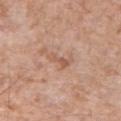Captured during whole-body skin photography for melanoma surveillance; the lesion was not biopsied.
A roughly 15 mm field-of-view crop from a total-body skin photograph.
An algorithmic analysis of the crop reported a footprint of about 3.5 mm², an outline eccentricity of about 0.85 (0 = round, 1 = elongated), and a symmetry-axis asymmetry near 0.5. And it measured radial color variation of about 0. And it measured a nevus-likeness score of about 0/100 and lesion-presence confidence of about 100/100.
On the right upper arm.
The lesion's longest dimension is about 3 mm.
The patient is a male roughly 55 years of age.
This is a white-light tile.